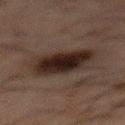| key | value |
|---|---|
| workup | catalogued during a skin exam; not biopsied |
| patient | male, aged approximately 50 |
| tile lighting | cross-polarized |
| location | the mid back |
| acquisition | 15 mm crop, total-body photography |